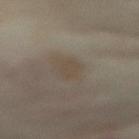This lesion was catalogued during total-body skin photography and was not selected for biopsy. The tile uses cross-polarized illumination. About 2.5 mm across. An algorithmic analysis of the crop reported a normalized lesion–skin contrast near 5. And it measured a border-irregularity rating of about 3/10 and a color-variation rating of about 1/10. It also reported a classifier nevus-likeness of about 5/100 and a detector confidence of about 90 out of 100 that the crop contains a lesion. A 15 mm close-up extracted from a 3D total-body photography capture. From the abdomen. The subject is a female in their mid-50s.